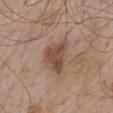{
  "biopsy_status": "not biopsied; imaged during a skin examination",
  "automated_metrics": {
    "cielab_L": 49,
    "cielab_a": 18,
    "cielab_b": 26,
    "vs_skin_darker_L": 10.0,
    "vs_skin_contrast_norm": 7.5,
    "border_irregularity_0_10": 3.0,
    "peripheral_color_asymmetry": 1.5,
    "nevus_likeness_0_100": 60
  },
  "patient": {
    "sex": "male",
    "age_approx": 55
  },
  "lesion_size": {
    "long_diameter_mm_approx": 4.5
  },
  "lighting": "white-light",
  "image": {
    "source": "total-body photography crop",
    "field_of_view_mm": 15
  },
  "site": "mid back"
}